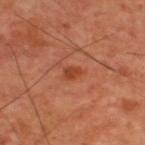notes: total-body-photography surveillance lesion; no biopsy
site: the upper back
lighting: cross-polarized
image: 15 mm crop, total-body photography
image-analysis metrics: roughly 8 lightness units darker than nearby skin and a normalized lesion–skin contrast near 7; a border-irregularity index near 2/10, a color-variation rating of about 2/10, and radial color variation of about 0.5; a nevus-likeness score of about 80/100 and a lesion-detection confidence of about 100/100
patient: male, approximately 60 years of age